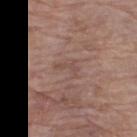Q: Is there a histopathology result?
A: total-body-photography surveillance lesion; no biopsy
Q: Automated lesion metrics?
A: a shape eccentricity near 0.95 and a symmetry-axis asymmetry near 0.6; a border-irregularity rating of about 7/10, a color-variation rating of about 0/10, and radial color variation of about 0
Q: What are the patient's age and sex?
A: female, aged around 70
Q: Lesion size?
A: about 3 mm
Q: What kind of image is this?
A: 15 mm crop, total-body photography
Q: Where on the body is the lesion?
A: the right thigh
Q: Illumination type?
A: white-light illumination